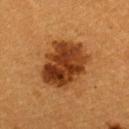Case summary:
– follow-up: no biopsy performed (imaged during a skin exam)
– anatomic site: the upper back
– TBP lesion metrics: a lesion area of about 24 mm², an eccentricity of roughly 0.6, and a shape-asymmetry score of about 0.15 (0 = symmetric); an average lesion color of about L≈33 a*≈23 b*≈34 (CIELAB), roughly 14 lightness units darker than nearby skin, and a normalized lesion–skin contrast near 11.5; a border-irregularity index near 2/10, a color-variation rating of about 6/10, and peripheral color asymmetry of about 2.5; a nevus-likeness score of about 100/100
– subject: female, roughly 55 years of age
– imaging modality: 15 mm crop, total-body photography
– diameter: about 6 mm